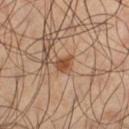Case summary:
• notes: catalogued during a skin exam; not biopsied
• location: the left thigh
• TBP lesion metrics: an average lesion color of about L≈48 a*≈20 b*≈32 (CIELAB), about 11 CIELAB-L* units darker than the surrounding skin, and a lesion-to-skin contrast of about 8.5 (normalized; higher = more distinct)
• subject: male, aged 58 to 62
• image source: 15 mm crop, total-body photography
• size: about 2.5 mm